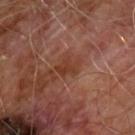Q: Was this lesion biopsied?
A: catalogued during a skin exam; not biopsied
Q: What are the patient's age and sex?
A: male, aged approximately 60
Q: Where on the body is the lesion?
A: the chest
Q: What did automated image analysis measure?
A: a mean CIELAB color near L≈36 a*≈23 b*≈29, roughly 7 lightness units darker than nearby skin, and a lesion-to-skin contrast of about 6.5 (normalized; higher = more distinct); border irregularity of about 4 on a 0–10 scale, a within-lesion color-variation index near 2/10, and peripheral color asymmetry of about 0.5; an automated nevus-likeness rating near 0 out of 100 and a lesion-detection confidence of about 100/100
Q: Illumination type?
A: cross-polarized illumination
Q: How was this image acquired?
A: 15 mm crop, total-body photography
Q: How large is the lesion?
A: ~2.5 mm (longest diameter)